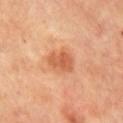{"biopsy_status": "not biopsied; imaged during a skin examination", "site": "chest", "automated_metrics": {"vs_skin_darker_L": 10.0, "vs_skin_contrast_norm": 7.0, "nevus_likeness_0_100": 80, "lesion_detection_confidence_0_100": 100}, "lesion_size": {"long_diameter_mm_approx": 3.5}, "image": {"source": "total-body photography crop", "field_of_view_mm": 15}, "lighting": "cross-polarized", "patient": {"sex": "male", "age_approx": 70}}